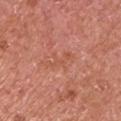Findings:
- notes — no biopsy performed (imaged during a skin exam)
- image — total-body-photography crop, ~15 mm field of view
- lighting — white-light
- patient — male, aged 63 to 67
- site — the right upper arm